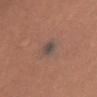workup = no biopsy performed (imaged during a skin exam)
subject = female, aged around 35
TBP lesion metrics = an average lesion color of about L≈46 a*≈13 b*≈19 (CIELAB) and a normalized lesion–skin contrast near 9; border irregularity of about 2 on a 0–10 scale, a within-lesion color-variation index near 3.5/10, and a peripheral color-asymmetry measure near 1; a nevus-likeness score of about 0/100 and a detector confidence of about 90 out of 100 that the crop contains a lesion
location = the upper back
lighting = white-light
size = ~3 mm (longest diameter)
imaging modality = ~15 mm crop, total-body skin-cancer survey